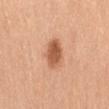<record>
<biopsy_status>not biopsied; imaged during a skin examination</biopsy_status>
<patient>
  <sex>female</sex>
  <age_approx>45</age_approx>
</patient>
<site>abdomen</site>
<image>
  <source>total-body photography crop</source>
  <field_of_view_mm>15</field_of_view_mm>
</image>
</record>